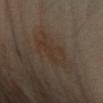Assessment: This lesion was catalogued during total-body skin photography and was not selected for biopsy. Background: The patient is a female aged 43–47. A region of skin cropped from a whole-body photographic capture, roughly 15 mm wide. This is a cross-polarized tile. An algorithmic analysis of the crop reported a color-variation rating of about 2.5/10 and peripheral color asymmetry of about 1. Located on the arm.